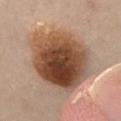Recorded during total-body skin imaging; not selected for excision or biopsy.
A male patient, about 50 years old.
The recorded lesion diameter is about 8 mm.
Automated tile analysis of the lesion measured an area of roughly 45 mm² and a shape-asymmetry score of about 0.15 (0 = symmetric). The analysis additionally found a lesion-detection confidence of about 100/100.
From the abdomen.
This is a cross-polarized tile.
Cropped from a whole-body photographic skin survey; the tile spans about 15 mm.